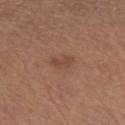Impression:
The lesion was photographed on a routine skin check and not biopsied; there is no pathology result.
Image and clinical context:
The patient is a male in their mid-60s. This image is a 15 mm lesion crop taken from a total-body photograph. On the left forearm. Longest diameter approximately 2.5 mm. Imaged with white-light lighting.